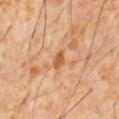| key | value |
|---|---|
| notes | total-body-photography surveillance lesion; no biopsy |
| diameter | ~2.5 mm (longest diameter) |
| subject | male, aged 58 to 62 |
| image source | 15 mm crop, total-body photography |
| body site | the front of the torso |
| illumination | cross-polarized |